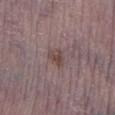{
  "biopsy_status": "not biopsied; imaged during a skin examination",
  "image": {
    "source": "total-body photography crop",
    "field_of_view_mm": 15
  },
  "site": "left lower leg",
  "automated_metrics": {
    "nevus_likeness_0_100": 10,
    "lesion_detection_confidence_0_100": 100
  },
  "patient": {
    "sex": "male",
    "age_approx": 75
  },
  "lighting": "white-light"
}